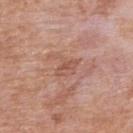<lesion>
  <biopsy_status>not biopsied; imaged during a skin examination</biopsy_status>
  <site>upper back</site>
  <lesion_size>
    <long_diameter_mm_approx>3.0</long_diameter_mm_approx>
  </lesion_size>
  <image>
    <source>total-body photography crop</source>
    <field_of_view_mm>15</field_of_view_mm>
  </image>
  <patient>
    <sex>male</sex>
    <age_approx>75</age_approx>
  </patient>
</lesion>